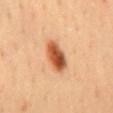Impression: Part of a total-body skin-imaging series; this lesion was reviewed on a skin check and was not flagged for biopsy. Acquisition and patient details: Longest diameter approximately 4.5 mm. Located on the mid back. The lesion-visualizer software estimated an outline eccentricity of about 0.85 (0 = round, 1 = elongated) and a shape-asymmetry score of about 0.2 (0 = symmetric). And it measured a lesion color around L≈57 a*≈29 b*≈40 in CIELAB, roughly 18 lightness units darker than nearby skin, and a lesion-to-skin contrast of about 11.5 (normalized; higher = more distinct). The software also gave a nevus-likeness score of about 100/100 and a detector confidence of about 100 out of 100 that the crop contains a lesion. Cropped from a total-body skin-imaging series; the visible field is about 15 mm. A male subject aged 38–42. The tile uses cross-polarized illumination.